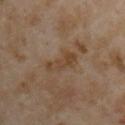patient — male, aged approximately 55
automated metrics — a lesion color around L≈43 a*≈16 b*≈30 in CIELAB, about 7 CIELAB-L* units darker than the surrounding skin, and a normalized lesion–skin contrast near 7; border irregularity of about 4.5 on a 0–10 scale, a within-lesion color-variation index near 3.5/10, and radial color variation of about 1; a classifier nevus-likeness of about 0/100 and lesion-presence confidence of about 100/100
body site — the right upper arm
diameter — ~4.5 mm (longest diameter)
image — total-body-photography crop, ~15 mm field of view
illumination — cross-polarized illumination